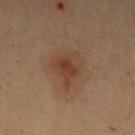biopsy status = catalogued during a skin exam; not biopsied | body site = the left upper arm | size = about 4.5 mm | lighting = cross-polarized | image source = ~15 mm crop, total-body skin-cancer survey | subject = male, in their 60s.